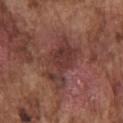* follow-up — catalogued during a skin exam; not biopsied
* acquisition — total-body-photography crop, ~15 mm field of view
* size — ~6 mm (longest diameter)
* body site — the chest
* subject — male, aged around 75